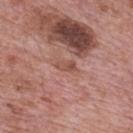workup — imaged on a skin check; not biopsied
site — the mid back
patient — male, about 70 years old
image — ~15 mm crop, total-body skin-cancer survey
TBP lesion metrics — a footprint of about 3 mm², an outline eccentricity of about 0.85 (0 = round, 1 = elongated), and a symmetry-axis asymmetry near 0.25